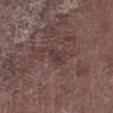Clinical impression:
The lesion was tiled from a total-body skin photograph and was not biopsied.
Acquisition and patient details:
Imaged with white-light lighting. From the right lower leg. A 15 mm close-up extracted from a 3D total-body photography capture. The subject is a male aged 73 to 77. Approximately 2.5 mm at its widest.